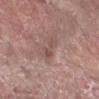{
  "patient": {
    "sex": "male",
    "age_approx": 65
  },
  "lighting": "white-light",
  "site": "right forearm",
  "automated_metrics": {
    "eccentricity": 0.95,
    "shape_asymmetry": 0.4,
    "cielab_L": 50,
    "cielab_a": 18,
    "cielab_b": 22,
    "vs_skin_darker_L": 8.0,
    "nevus_likeness_0_100": 0,
    "lesion_detection_confidence_0_100": 90
  },
  "image": {
    "source": "total-body photography crop",
    "field_of_view_mm": 15
  },
  "lesion_size": {
    "long_diameter_mm_approx": 3.5
  }
}